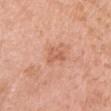A 15 mm crop from a total-body photograph taken for skin-cancer surveillance.
The tile uses white-light illumination.
The patient is a female aged 48–52.
From the head or neck.
About 3 mm across.